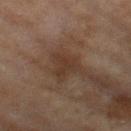image source: total-body-photography crop, ~15 mm field of view
subject: female, aged around 60
location: the left thigh